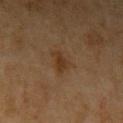follow-up: imaged on a skin check; not biopsied | lighting: cross-polarized illumination | patient: female, approximately 70 years of age | diameter: ~3 mm (longest diameter) | site: the left upper arm | automated lesion analysis: a lesion area of about 6 mm², an outline eccentricity of about 0.55 (0 = round, 1 = elongated), and a symmetry-axis asymmetry near 0.35; an average lesion color of about L≈30 a*≈14 b*≈27 (CIELAB); border irregularity of about 3.5 on a 0–10 scale, internal color variation of about 2.5 on a 0–10 scale, and a peripheral color-asymmetry measure near 1; a classifier nevus-likeness of about 35/100 and a lesion-detection confidence of about 100/100 | image source: ~15 mm tile from a whole-body skin photo.